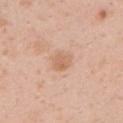The lesion was tiled from a total-body skin photograph and was not biopsied. This is a white-light tile. The lesion's longest dimension is about 3 mm. From the left upper arm. A lesion tile, about 15 mm wide, cut from a 3D total-body photograph. A male patient in their 30s.